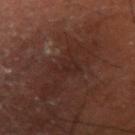Recorded during total-body skin imaging; not selected for excision or biopsy. Automated tile analysis of the lesion measured a mean CIELAB color near L≈22 a*≈17 b*≈19 and a normalized lesion–skin contrast near 5. It also reported an automated nevus-likeness rating near 0 out of 100. On the right upper arm. This is a cross-polarized tile. A 15 mm close-up extracted from a 3D total-body photography capture. A male subject, in their mid-50s. Longest diameter approximately 3 mm.